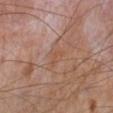Assessment: Captured during whole-body skin photography for melanoma surveillance; the lesion was not biopsied. Background: A 15 mm crop from a total-body photograph taken for skin-cancer surveillance. The lesion-visualizer software estimated an area of roughly 2.5 mm² and an outline eccentricity of about 0.85 (0 = round, 1 = elongated). And it measured a lesion color around L≈49 a*≈20 b*≈29 in CIELAB, a lesion–skin lightness drop of about 4, and a normalized lesion–skin contrast near 5. Measured at roughly 2.5 mm in maximum diameter. Located on the left lower leg. A male patient approximately 65 years of age. The tile uses cross-polarized illumination.